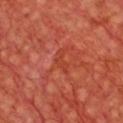This lesion was catalogued during total-body skin photography and was not selected for biopsy.
A close-up tile cropped from a whole-body skin photograph, about 15 mm across.
A male patient in their mid- to late 60s.
The lesion is located on the chest.
Captured under cross-polarized illumination.
An algorithmic analysis of the crop reported a mean CIELAB color near L≈44 a*≈37 b*≈37, roughly 5 lightness units darker than nearby skin, and a normalized border contrast of about 5.